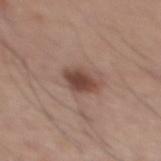* follow-up · imaged on a skin check; not biopsied
* diameter · about 3.5 mm
* lighting · white-light
* acquisition · total-body-photography crop, ~15 mm field of view
* patient · male, about 30 years old
* site · the mid back